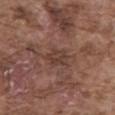Case summary:
* biopsy status: total-body-photography surveillance lesion; no biopsy
* size: ~3 mm (longest diameter)
* subject: male, in their mid- to late 70s
* imaging modality: 15 mm crop, total-body photography
* automated lesion analysis: a footprint of about 5.5 mm², a shape eccentricity near 0.7, and two-axis asymmetry of about 0.25; a lesion color around L≈39 a*≈18 b*≈23 in CIELAB, roughly 7 lightness units darker than nearby skin, and a lesion-to-skin contrast of about 6 (normalized; higher = more distinct)
* anatomic site: the abdomen